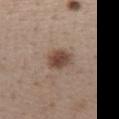Acquisition and patient details:
Captured under white-light illumination. This image is a 15 mm lesion crop taken from a total-body photograph. The lesion is on the chest. Automated image analysis of the tile measured an average lesion color of about L≈46 a*≈17 b*≈25 (CIELAB), a lesion–skin lightness drop of about 12, and a normalized border contrast of about 9. The analysis additionally found border irregularity of about 1.5 on a 0–10 scale and a within-lesion color-variation index near 4.5/10. Approximately 3 mm at its widest. A female patient, in their 40s.